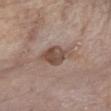From the chest. A 15 mm close-up tile from a total-body photography series done for melanoma screening. A male patient approximately 65 years of age.